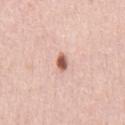Impression:
Captured during whole-body skin photography for melanoma surveillance; the lesion was not biopsied.
Context:
A male subject roughly 40 years of age. This is a white-light tile. About 2.5 mm across. From the chest. A 15 mm crop from a total-body photograph taken for skin-cancer surveillance.